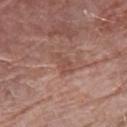| key | value |
|---|---|
| follow-up | imaged on a skin check; not biopsied |
| imaging modality | 15 mm crop, total-body photography |
| body site | the right forearm |
| image-analysis metrics | a normalized border contrast of about 5; a color-variation rating of about 1/10; an automated nevus-likeness rating near 0 out of 100 and a detector confidence of about 100 out of 100 that the crop contains a lesion |
| lighting | white-light illumination |
| diameter | ≈3 mm |
| subject | female, approximately 65 years of age |